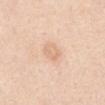| field | value |
|---|---|
| notes | catalogued during a skin exam; not biopsied |
| illumination | white-light illumination |
| patient | female, in their mid-40s |
| site | the chest |
| lesion diameter | about 3 mm |
| automated lesion analysis | a lesion color around L≈73 a*≈19 b*≈33 in CIELAB, about 7 CIELAB-L* units darker than the surrounding skin, and a normalized lesion–skin contrast near 4.5; a nevus-likeness score of about 0/100 and a lesion-detection confidence of about 100/100 |
| imaging modality | ~15 mm crop, total-body skin-cancer survey |